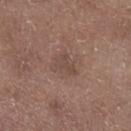Impression:
No biopsy was performed on this lesion — it was imaged during a full skin examination and was not determined to be concerning.
Context:
A male patient, about 70 years old. The recorded lesion diameter is about 3 mm. A roughly 15 mm field-of-view crop from a total-body skin photograph. The tile uses white-light illumination. The lesion-visualizer software estimated an average lesion color of about L≈46 a*≈17 b*≈23 (CIELAB). It also reported a within-lesion color-variation index near 1.5/10 and a peripheral color-asymmetry measure near 0.5. It also reported a lesion-detection confidence of about 100/100. Located on the left lower leg.